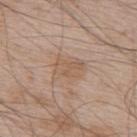Assessment: No biopsy was performed on this lesion — it was imaged during a full skin examination and was not determined to be concerning. Context: On the upper back. The total-body-photography lesion software estimated an outline eccentricity of about 0.7 (0 = round, 1 = elongated) and two-axis asymmetry of about 0.3. The software also gave a lesion color around L≈57 a*≈16 b*≈30 in CIELAB, about 6 CIELAB-L* units darker than the surrounding skin, and a lesion-to-skin contrast of about 5.5 (normalized; higher = more distinct). A male subject, in their mid- to late 60s. A region of skin cropped from a whole-body photographic capture, roughly 15 mm wide. Measured at roughly 3.5 mm in maximum diameter. The tile uses white-light illumination.